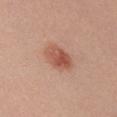Recorded during total-body skin imaging; not selected for excision or biopsy. Cropped from a whole-body photographic skin survey; the tile spans about 15 mm. The subject is a female aged approximately 25. Located on the right upper arm.